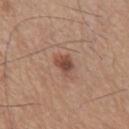<record>
<biopsy_status>not biopsied; imaged during a skin examination</biopsy_status>
<image>
  <source>total-body photography crop</source>
  <field_of_view_mm>15</field_of_view_mm>
</image>
<lesion_size>
  <long_diameter_mm_approx>2.5</long_diameter_mm_approx>
</lesion_size>
<automated_metrics>
  <nevus_likeness_0_100>90</nevus_likeness_0_100>
</automated_metrics>
<site>mid back</site>
<patient>
  <sex>male</sex>
  <age_approx>60</age_approx>
</patient>
<lighting>white-light</lighting>
</record>